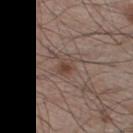Imaged during a routine full-body skin examination; the lesion was not biopsied and no histopathology is available.
Longest diameter approximately 3 mm.
Automated image analysis of the tile measured a border-irregularity rating of about 3/10, internal color variation of about 3 on a 0–10 scale, and peripheral color asymmetry of about 1. The software also gave a classifier nevus-likeness of about 30/100 and a detector confidence of about 100 out of 100 that the crop contains a lesion.
The subject is a male roughly 60 years of age.
This is a white-light tile.
A 15 mm close-up extracted from a 3D total-body photography capture.
Located on the right lower leg.